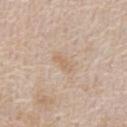follow-up = catalogued during a skin exam; not biopsied | patient = female, roughly 60 years of age | acquisition = ~15 mm tile from a whole-body skin photo | lighting = white-light illumination | body site = the chest.